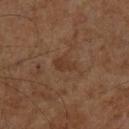Assessment: The lesion was tiled from a total-body skin photograph and was not biopsied. Acquisition and patient details: The lesion is located on the left lower leg. Measured at roughly 3 mm in maximum diameter. A region of skin cropped from a whole-body photographic capture, roughly 15 mm wide. The tile uses cross-polarized illumination. Automated image analysis of the tile measured about 5 CIELAB-L* units darker than the surrounding skin and a normalized lesion–skin contrast near 5.5. And it measured border irregularity of about 3 on a 0–10 scale and internal color variation of about 1 on a 0–10 scale. A male patient aged around 60.